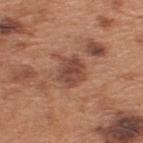Findings:
• notes · imaged on a skin check; not biopsied
• patient · male, aged approximately 65
• image source · total-body-photography crop, ~15 mm field of view
• site · the back